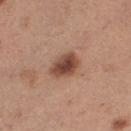workup — catalogued during a skin exam; not biopsied
image source — total-body-photography crop, ~15 mm field of view
lesion size — ~3.5 mm (longest diameter)
body site — the leg
patient — female, aged 28–32
tile lighting — white-light